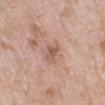Findings:
* location: the left lower leg
* patient: female, aged approximately 70
* lighting: white-light
* acquisition: ~15 mm crop, total-body skin-cancer survey
* diameter: ≈2.5 mm